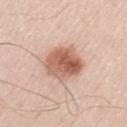notes: total-body-photography surveillance lesion; no biopsy
patient: male, in their 70s
image source: ~15 mm crop, total-body skin-cancer survey
lesion size: about 5 mm
lighting: white-light
site: the left thigh